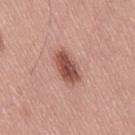Q: Is there a histopathology result?
A: no biopsy performed (imaged during a skin exam)
Q: How was this image acquired?
A: total-body-photography crop, ~15 mm field of view
Q: What is the lesion's diameter?
A: about 4 mm
Q: What are the patient's age and sex?
A: male, in their mid-50s
Q: Where on the body is the lesion?
A: the right thigh
Q: How was the tile lit?
A: white-light